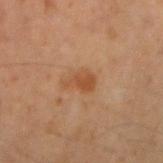The lesion was tiled from a total-body skin photograph and was not biopsied. The total-body-photography lesion software estimated a border-irregularity index near 4/10, internal color variation of about 2 on a 0–10 scale, and peripheral color asymmetry of about 0.5. A lesion tile, about 15 mm wide, cut from a 3D total-body photograph. A male patient, aged approximately 50. The lesion's longest dimension is about 3.5 mm. Imaged with cross-polarized lighting. On the right upper arm.